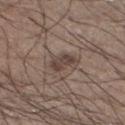Clinical impression: Imaged during a routine full-body skin examination; the lesion was not biopsied and no histopathology is available. Context: A 15 mm close-up extracted from a 3D total-body photography capture. This is a white-light tile. A male subject approximately 35 years of age. On the left lower leg.